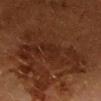No biopsy was performed on this lesion — it was imaged during a full skin examination and was not determined to be concerning.
A female subject, in their 50s.
Automated image analysis of the tile measured a footprint of about 4 mm² and an outline eccentricity of about 0.85 (0 = round, 1 = elongated). And it measured a nevus-likeness score of about 0/100 and lesion-presence confidence of about 85/100.
A roughly 15 mm field-of-view crop from a total-body skin photograph.
Located on the chest.
This is a cross-polarized tile.